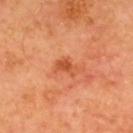Q: Was a biopsy performed?
A: no biopsy performed (imaged during a skin exam)
Q: What are the patient's age and sex?
A: male, in their mid- to late 60s
Q: Automated lesion metrics?
A: a lesion color around L≈54 a*≈33 b*≈42 in CIELAB; border irregularity of about 3.5 on a 0–10 scale and a within-lesion color-variation index near 2.5/10; an automated nevus-likeness rating near 20 out of 100 and lesion-presence confidence of about 100/100
Q: What kind of image is this?
A: ~15 mm crop, total-body skin-cancer survey
Q: What is the anatomic site?
A: the upper back